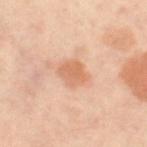| key | value |
|---|---|
| notes | total-body-photography surveillance lesion; no biopsy |
| location | the right thigh |
| image source | total-body-photography crop, ~15 mm field of view |
| automated lesion analysis | a lesion color around L≈67 a*≈24 b*≈36 in CIELAB, about 10 CIELAB-L* units darker than the surrounding skin, and a normalized lesion–skin contrast near 6.5; border irregularity of about 2.5 on a 0–10 scale, a within-lesion color-variation index near 2.5/10, and peripheral color asymmetry of about 1 |
| size | ≈3 mm |
| tile lighting | cross-polarized |
| patient | female, roughly 50 years of age |